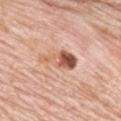Assessment:
This lesion was catalogued during total-body skin photography and was not selected for biopsy.